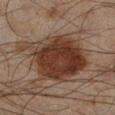Findings:
* notes — catalogued during a skin exam; not biopsied
* patient — male, in their mid- to late 40s
* location — the leg
* image — total-body-photography crop, ~15 mm field of view